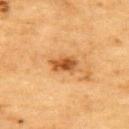Assessment:
Recorded during total-body skin imaging; not selected for excision or biopsy.
Background:
Automated tile analysis of the lesion measured a mean CIELAB color near L≈49 a*≈23 b*≈40, roughly 12 lightness units darker than nearby skin, and a normalized lesion–skin contrast near 9. The software also gave a border-irregularity rating of about 2.5/10, a within-lesion color-variation index near 4.5/10, and peripheral color asymmetry of about 1.5. The software also gave a classifier nevus-likeness of about 85/100. From the upper back. A male subject aged 83 or over. The lesion's longest dimension is about 3.5 mm. A 15 mm close-up extracted from a 3D total-body photography capture.